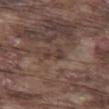Q: Is there a histopathology result?
A: no biopsy performed (imaged during a skin exam)
Q: What kind of image is this?
A: 15 mm crop, total-body photography
Q: Who is the patient?
A: male, aged around 75
Q: How large is the lesion?
A: ≈2.5 mm
Q: What is the anatomic site?
A: the left thigh